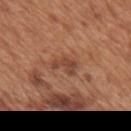workup = no biopsy performed (imaged during a skin exam); subject = male, in their mid- to late 60s; image = 15 mm crop, total-body photography; location = the mid back; diameter = about 3 mm; tile lighting = white-light.